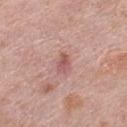Q: Is there a histopathology result?
A: no biopsy performed (imaged during a skin exam)
Q: How large is the lesion?
A: about 2.5 mm
Q: Where on the body is the lesion?
A: the left thigh
Q: How was this image acquired?
A: total-body-photography crop, ~15 mm field of view
Q: What are the patient's age and sex?
A: female, aged 38–42